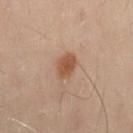The lesion was photographed on a routine skin check and not biopsied; there is no pathology result. The tile uses cross-polarized illumination. The lesion is located on the back. A lesion tile, about 15 mm wide, cut from a 3D total-body photograph. An algorithmic analysis of the crop reported a nevus-likeness score of about 95/100 and a lesion-detection confidence of about 100/100. A male patient aged 48 to 52.